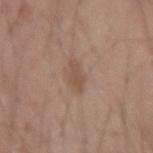Q: What kind of image is this?
A: 15 mm crop, total-body photography
Q: Patient demographics?
A: male, aged approximately 20
Q: Lesion location?
A: the right forearm